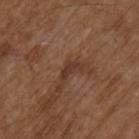This is a white-light tile.
Located on the right upper arm.
This image is a 15 mm lesion crop taken from a total-body photograph.
The subject is a male approximately 75 years of age.